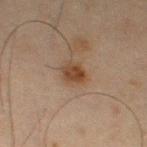Notes:
- lighting · cross-polarized illumination
- size · about 2.5 mm
- imaging modality · ~15 mm tile from a whole-body skin photo
- patient · male, aged 63–67
- location · the left thigh